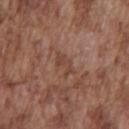On the chest.
A male patient aged 73 to 77.
A 15 mm close-up tile from a total-body photography series done for melanoma screening.
Automated tile analysis of the lesion measured a lesion color around L≈43 a*≈21 b*≈27 in CIELAB, roughly 7 lightness units darker than nearby skin, and a normalized lesion–skin contrast near 6. The analysis additionally found a border-irregularity index near 5/10 and a color-variation rating of about 0.5/10. The software also gave a classifier nevus-likeness of about 0/100.
This is a white-light tile.